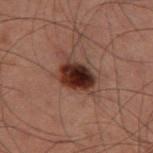Case summary:
– follow-up — no biopsy performed (imaged during a skin exam)
– lesion diameter — about 4 mm
– site — the left thigh
– image source — ~15 mm tile from a whole-body skin photo
– patient — male, aged 58–62
– lighting — cross-polarized